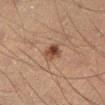{
  "biopsy_status": "not biopsied; imaged during a skin examination",
  "site": "left lower leg",
  "automated_metrics": {
    "area_mm2_approx": 4.5,
    "eccentricity": 0.6,
    "shape_asymmetry": 0.2,
    "vs_skin_contrast_norm": 9.0,
    "border_irregularity_0_10": 2.0,
    "peripheral_color_asymmetry": 2.5,
    "nevus_likeness_0_100": 95,
    "lesion_detection_confidence_0_100": 100
  },
  "lighting": "cross-polarized",
  "image": {
    "source": "total-body photography crop",
    "field_of_view_mm": 15
  },
  "lesion_size": {
    "long_diameter_mm_approx": 2.5
  },
  "patient": {
    "sex": "male",
    "age_approx": 40
  }
}